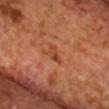Findings:
* follow-up · no biopsy performed (imaged during a skin exam)
* site · the chest
* diameter · about 2.5 mm
* patient · male, roughly 70 years of age
* TBP lesion metrics · a footprint of about 3 mm² and an outline eccentricity of about 0.85 (0 = round, 1 = elongated); a mean CIELAB color near L≈44 a*≈27 b*≈37, roughly 7 lightness units darker than nearby skin, and a normalized border contrast of about 6
* image · total-body-photography crop, ~15 mm field of view